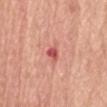  biopsy_status: not biopsied; imaged during a skin examination